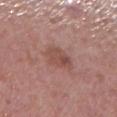Case summary:
- image source: ~15 mm tile from a whole-body skin photo
- site: the right lower leg
- patient: female, aged around 40
- size: ≈3.5 mm
- tile lighting: white-light illumination